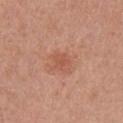biopsy status = no biopsy performed (imaged during a skin exam); site = the left upper arm; acquisition = total-body-photography crop, ~15 mm field of view; illumination = white-light; patient = female, about 55 years old.